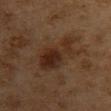biopsy status: catalogued during a skin exam; not biopsied | lesion size: about 7 mm | body site: the chest | tile lighting: cross-polarized illumination | image source: ~15 mm tile from a whole-body skin photo | patient: female, about 60 years old.